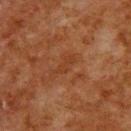Assessment:
Imaged during a routine full-body skin examination; the lesion was not biopsied and no histopathology is available.
Image and clinical context:
Longest diameter approximately 3 mm. Automated tile analysis of the lesion measured a detector confidence of about 100 out of 100 that the crop contains a lesion. A 15 mm close-up extracted from a 3D total-body photography capture. Captured under cross-polarized illumination. The subject is a male approximately 80 years of age. Located on the upper back.